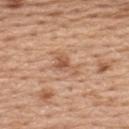The lesion was tiled from a total-body skin photograph and was not biopsied.
About 3.5 mm across.
The patient is a female approximately 55 years of age.
From the upper back.
This is a white-light tile.
A roughly 15 mm field-of-view crop from a total-body skin photograph.